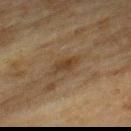Captured during whole-body skin photography for melanoma surveillance; the lesion was not biopsied.
A 15 mm close-up tile from a total-body photography series done for melanoma screening.
About 3.5 mm across.
The lesion is on the upper back.
A male patient, in their 70s.
Imaged with cross-polarized lighting.